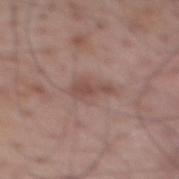The lesion was photographed on a routine skin check and not biopsied; there is no pathology result.
An algorithmic analysis of the crop reported a lesion color around L≈49 a*≈19 b*≈23 in CIELAB, a lesion–skin lightness drop of about 8, and a normalized lesion–skin contrast near 6.
Approximately 4 mm at its widest.
Captured under white-light illumination.
Cropped from a whole-body photographic skin survey; the tile spans about 15 mm.
From the mid back.
A male subject aged 68–72.